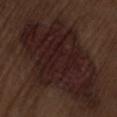Captured during whole-body skin photography for melanoma surveillance; the lesion was not biopsied. On the back. This image is a 15 mm lesion crop taken from a total-body photograph. This is a white-light tile. Approximately 16.5 mm at its widest. The subject is a male in their 70s.